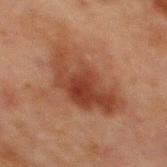Clinical impression:
Recorded during total-body skin imaging; not selected for excision or biopsy.
Clinical summary:
On the chest. A close-up tile cropped from a whole-body skin photograph, about 15 mm across. Imaged with cross-polarized lighting. Automated tile analysis of the lesion measured a footprint of about 25 mm², a shape eccentricity near 0.85, and a shape-asymmetry score of about 0.35 (0 = symmetric). The analysis additionally found a border-irregularity index near 4/10, internal color variation of about 6 on a 0–10 scale, and radial color variation of about 2. The analysis additionally found a nevus-likeness score of about 15/100 and lesion-presence confidence of about 100/100. A male patient, about 65 years old. Measured at roughly 8 mm in maximum diameter.